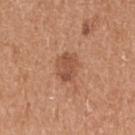Case summary:
– follow-up: catalogued during a skin exam; not biopsied
– patient: female, aged 33 to 37
– site: the arm
– imaging modality: total-body-photography crop, ~15 mm field of view